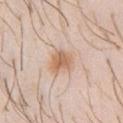follow-up: total-body-photography surveillance lesion; no biopsy | lesion size: ≈3 mm | illumination: white-light | anatomic site: the chest | image: total-body-photography crop, ~15 mm field of view | patient: male, approximately 30 years of age.